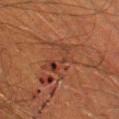workup — imaged on a skin check; not biopsied
anatomic site — the left lower leg
patient — male, aged 58–62
acquisition — ~15 mm tile from a whole-body skin photo